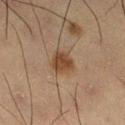From the left thigh. An algorithmic analysis of the crop reported a border-irregularity index near 1.5/10, a color-variation rating of about 3/10, and peripheral color asymmetry of about 1. It also reported a lesion-detection confidence of about 100/100. Captured under cross-polarized illumination. A roughly 15 mm field-of-view crop from a total-body skin photograph. A male subject aged 53–57.